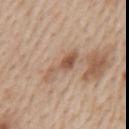From the mid back.
Cropped from a total-body skin-imaging series; the visible field is about 15 mm.
This is a white-light tile.
About 5 mm across.
A male subject approximately 60 years of age.
Automated image analysis of the tile measured a lesion area of about 5.5 mm² and an eccentricity of roughly 0.95.